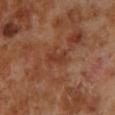Assessment:
Captured during whole-body skin photography for melanoma surveillance; the lesion was not biopsied.
Acquisition and patient details:
A male patient roughly 70 years of age. A 15 mm close-up extracted from a 3D total-body photography capture. The tile uses cross-polarized illumination. From the left lower leg. Automated tile analysis of the lesion measured a footprint of about 3 mm² and a shape-asymmetry score of about 0.35 (0 = symmetric). And it measured a border-irregularity rating of about 3.5/10 and radial color variation of about 0. Approximately 2.5 mm at its widest.